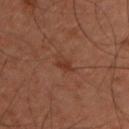Impression: The lesion was photographed on a routine skin check and not biopsied; there is no pathology result. Image and clinical context: The total-body-photography lesion software estimated an average lesion color of about L≈34 a*≈22 b*≈28 (CIELAB) and a normalized border contrast of about 6. The analysis additionally found an automated nevus-likeness rating near 5 out of 100 and a detector confidence of about 100 out of 100 that the crop contains a lesion. A male subject roughly 45 years of age. This image is a 15 mm lesion crop taken from a total-body photograph. Measured at roughly 2.5 mm in maximum diameter. From the back. Imaged with cross-polarized lighting.